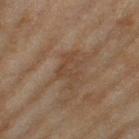tile lighting=cross-polarized | anatomic site=the left thigh | image source=~15 mm tile from a whole-body skin photo | size=~4 mm (longest diameter) | subject=female, aged approximately 60 | image-analysis metrics=an average lesion color of about L≈39 a*≈15 b*≈27 (CIELAB) and roughly 5 lightness units darker than nearby skin; a border-irregularity rating of about 6.5/10, a within-lesion color-variation index near 2/10, and peripheral color asymmetry of about 0.5; a nevus-likeness score of about 0/100.